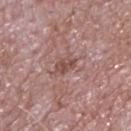{
  "biopsy_status": "not biopsied; imaged during a skin examination",
  "lighting": "white-light",
  "image": {
    "source": "total-body photography crop",
    "field_of_view_mm": 15
  },
  "site": "upper back",
  "patient": {
    "sex": "male",
    "age_approx": 65
  },
  "automated_metrics": {
    "vs_skin_contrast_norm": 7.0,
    "border_irregularity_0_10": 3.0,
    "color_variation_0_10": 2.5
  },
  "lesion_size": {
    "long_diameter_mm_approx": 2.5
  }
}